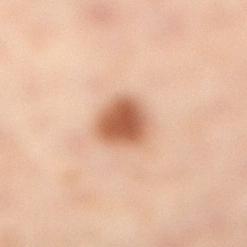biopsy_status: not biopsied; imaged during a skin examination
site: left leg
image:
  source: total-body photography crop
  field_of_view_mm: 15
lesion_size:
  long_diameter_mm_approx: 3.5
lighting: cross-polarized
patient:
  sex: female
  age_approx: 55